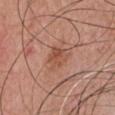Clinical impression:
The lesion was photographed on a routine skin check and not biopsied; there is no pathology result.
Background:
Longest diameter approximately 3.5 mm. A male subject in their 50s. The tile uses white-light illumination. Cropped from a total-body skin-imaging series; the visible field is about 15 mm. From the chest.